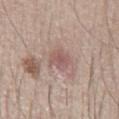Recorded during total-body skin imaging; not selected for excision or biopsy.
From the abdomen.
A male patient aged around 40.
A lesion tile, about 15 mm wide, cut from a 3D total-body photograph.
Automated tile analysis of the lesion measured an area of roughly 5.5 mm² and an eccentricity of roughly 0.55. The software also gave a lesion color around L≈55 a*≈19 b*≈21 in CIELAB, roughly 8 lightness units darker than nearby skin, and a normalized lesion–skin contrast near 6. The software also gave a border-irregularity index near 2.5/10, a color-variation rating of about 2.5/10, and radial color variation of about 1. It also reported a classifier nevus-likeness of about 40/100 and a detector confidence of about 100 out of 100 that the crop contains a lesion.
Approximately 3 mm at its widest.
This is a white-light tile.